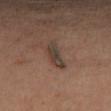The lesion was photographed on a routine skin check and not biopsied; there is no pathology result. A 15 mm crop from a total-body photograph taken for skin-cancer surveillance. Longest diameter approximately 4.5 mm. Located on the left leg. The total-body-photography lesion software estimated a border-irregularity rating of about 4/10, internal color variation of about 2.5 on a 0–10 scale, and a peripheral color-asymmetry measure near 0.5. The analysis additionally found a classifier nevus-likeness of about 0/100 and lesion-presence confidence of about 90/100. The patient is a male aged 48–52. This is a cross-polarized tile.